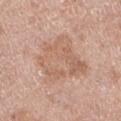Recorded during total-body skin imaging; not selected for excision or biopsy. Captured under white-light illumination. A female patient aged approximately 65. The lesion's longest dimension is about 6 mm. From the left thigh. A roughly 15 mm field-of-view crop from a total-body skin photograph. An algorithmic analysis of the crop reported a color-variation rating of about 4/10 and a peripheral color-asymmetry measure near 1.5. It also reported a nevus-likeness score of about 0/100 and a detector confidence of about 100 out of 100 that the crop contains a lesion.